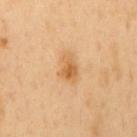Q: Was this lesion biopsied?
A: catalogued during a skin exam; not biopsied
Q: How was this image acquired?
A: ~15 mm tile from a whole-body skin photo
Q: How large is the lesion?
A: about 3.5 mm
Q: Where on the body is the lesion?
A: the mid back
Q: Who is the patient?
A: male, about 50 years old
Q: What lighting was used for the tile?
A: cross-polarized illumination
Q: Automated lesion metrics?
A: a footprint of about 6.5 mm², a shape eccentricity near 0.8, and two-axis asymmetry of about 0.3; a border-irregularity rating of about 3/10, a color-variation rating of about 5.5/10, and radial color variation of about 1.5